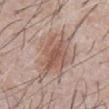notes: imaged on a skin check; not biopsied | image: 15 mm crop, total-body photography | patient: male, aged 58–62 | TBP lesion metrics: a footprint of about 23 mm² and two-axis asymmetry of about 0.25; a border-irregularity index near 4.5/10, a color-variation rating of about 5.5/10, and radial color variation of about 1.5; a classifier nevus-likeness of about 75/100 and lesion-presence confidence of about 100/100 | body site: the abdomen | lesion diameter: ~7 mm (longest diameter).